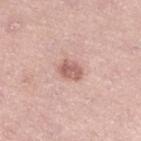Findings:
- workup — total-body-photography surveillance lesion; no biopsy
- lighting — white-light illumination
- automated metrics — a footprint of about 4.5 mm², a shape eccentricity near 0.7, and a symmetry-axis asymmetry near 0.25; about 12 CIELAB-L* units darker than the surrounding skin and a normalized border contrast of about 7.5; a classifier nevus-likeness of about 60/100
- patient — female, approximately 40 years of age
- lesion diameter — ~3 mm (longest diameter)
- site — the leg
- image — total-body-photography crop, ~15 mm field of view